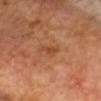Image and clinical context:
A 15 mm crop from a total-body photograph taken for skin-cancer surveillance. About 2.5 mm across. Located on the head or neck. A male patient, in their 70s. Automated image analysis of the tile measured a footprint of about 3 mm², an outline eccentricity of about 0.85 (0 = round, 1 = elongated), and a symmetry-axis asymmetry near 0.4. And it measured a border-irregularity rating of about 4/10 and a peripheral color-asymmetry measure near 0. The analysis additionally found an automated nevus-likeness rating near 0 out of 100 and a detector confidence of about 100 out of 100 that the crop contains a lesion.